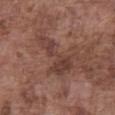{"biopsy_status": "not biopsied; imaged during a skin examination", "lesion_size": {"long_diameter_mm_approx": 6.0}, "lighting": "white-light", "site": "front of the torso", "image": {"source": "total-body photography crop", "field_of_view_mm": 15}, "patient": {"sex": "male", "age_approx": 75}}